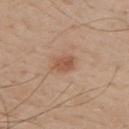{"biopsy_status": "not biopsied; imaged during a skin examination", "image": {"source": "total-body photography crop", "field_of_view_mm": 15}, "lesion_size": {"long_diameter_mm_approx": 2.5}, "patient": {"sex": "male", "age_approx": 70}, "automated_metrics": {"cielab_L": 54, "cielab_a": 22, "cielab_b": 31, "vs_skin_darker_L": 9.0, "vs_skin_contrast_norm": 6.5, "border_irregularity_0_10": 2.0, "peripheral_color_asymmetry": 1.0}, "site": "back", "lighting": "white-light"}